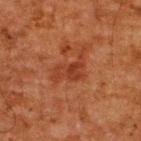Impression:
Recorded during total-body skin imaging; not selected for excision or biopsy.
Acquisition and patient details:
This image is a 15 mm lesion crop taken from a total-body photograph. Measured at roughly 4.5 mm in maximum diameter. The subject is a male aged approximately 60. On the upper back. This is a cross-polarized tile.